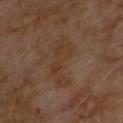biopsy status = total-body-photography surveillance lesion; no biopsy | lesion size = about 6 mm | anatomic site = the upper back | illumination = cross-polarized | automated lesion analysis = an area of roughly 11 mm² and two-axis asymmetry of about 0.55; a border-irregularity rating of about 8/10 and internal color variation of about 3 on a 0–10 scale; an automated nevus-likeness rating near 0 out of 100 and lesion-presence confidence of about 100/100 | subject = male, approximately 70 years of age | image = ~15 mm crop, total-body skin-cancer survey.